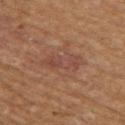{"biopsy_status": "not biopsied; imaged during a skin examination", "image": {"source": "total-body photography crop", "field_of_view_mm": 15}, "automated_metrics": {"cielab_L": 40, "cielab_a": 19, "cielab_b": 24, "vs_skin_darker_L": 6.0, "vs_skin_contrast_norm": 5.5, "border_irregularity_0_10": 4.0, "color_variation_0_10": 4.0}, "patient": {"sex": "male", "age_approx": 65}, "site": "left thigh", "lesion_size": {"long_diameter_mm_approx": 4.5}, "lighting": "cross-polarized"}